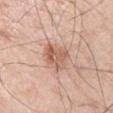workup = imaged on a skin check; not biopsied.